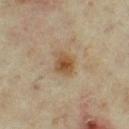Longest diameter approximately 3 mm.
This image is a 15 mm lesion crop taken from a total-body photograph.
The tile uses cross-polarized illumination.
The lesion is on the left thigh.
The patient is a female in their mid-30s.